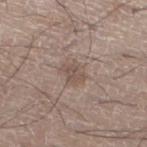  biopsy_status: not biopsied; imaged during a skin examination
  lesion_size:
    long_diameter_mm_approx: 3.0
  site: leg
  patient:
    sex: male
    age_approx: 75
  lighting: white-light
  image:
    source: total-body photography crop
    field_of_view_mm: 15